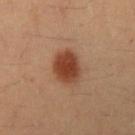Part of a total-body skin-imaging series; this lesion was reviewed on a skin check and was not flagged for biopsy.
The tile uses cross-polarized illumination.
On the arm.
The patient is a female aged approximately 35.
A 15 mm close-up tile from a total-body photography series done for melanoma screening.